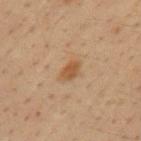{
  "biopsy_status": "not biopsied; imaged during a skin examination"
}